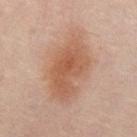Assessment: No biopsy was performed on this lesion — it was imaged during a full skin examination and was not determined to be concerning. Acquisition and patient details: Imaged with cross-polarized lighting. Located on the mid back. A close-up tile cropped from a whole-body skin photograph, about 15 mm across. The subject is a female aged 48–52. Approximately 9 mm at its widest.